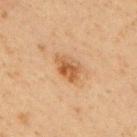Clinical impression:
Recorded during total-body skin imaging; not selected for excision or biopsy.
Clinical summary:
The recorded lesion diameter is about 3.5 mm. The tile uses cross-polarized illumination. The lesion-visualizer software estimated a lesion color around L≈48 a*≈19 b*≈34 in CIELAB, a lesion–skin lightness drop of about 9, and a normalized lesion–skin contrast near 7.5. The analysis additionally found a classifier nevus-likeness of about 70/100 and a detector confidence of about 100 out of 100 that the crop contains a lesion. A male subject aged approximately 60. This image is a 15 mm lesion crop taken from a total-body photograph. The lesion is located on the back.